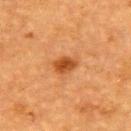notes: imaged on a skin check; not biopsied | subject: female, aged 53 to 57 | anatomic site: the upper back | image source: 15 mm crop, total-body photography.